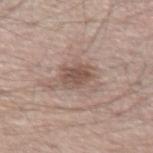The lesion was tiled from a total-body skin photograph and was not biopsied. A 15 mm close-up extracted from a 3D total-body photography capture. Automated tile analysis of the lesion measured a border-irregularity rating of about 2.5/10 and radial color variation of about 1. The analysis additionally found an automated nevus-likeness rating near 40 out of 100 and a detector confidence of about 100 out of 100 that the crop contains a lesion. The lesion is on the abdomen. The subject is a male aged 63–67. Approximately 3.5 mm at its widest.